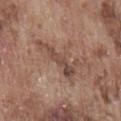Findings:
- biopsy status: no biopsy performed (imaged during a skin exam)
- lighting: white-light
- site: the lower back
- patient: male, aged 73–77
- acquisition: ~15 mm tile from a whole-body skin photo
- TBP lesion metrics: a border-irregularity index near 9/10 and internal color variation of about 3 on a 0–10 scale